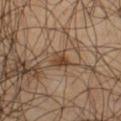Recorded during total-body skin imaging; not selected for excision or biopsy.